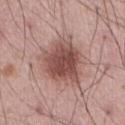The lesion was photographed on a routine skin check and not biopsied; there is no pathology result. Located on the front of the torso. The lesion-visualizer software estimated a mean CIELAB color near L≈51 a*≈22 b*≈23 and a lesion–skin lightness drop of about 13. The software also gave internal color variation of about 5 on a 0–10 scale and radial color variation of about 1.5. Measured at roughly 6 mm in maximum diameter. A male subject, aged around 55. A close-up tile cropped from a whole-body skin photograph, about 15 mm across. The tile uses white-light illumination.